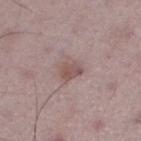Clinical impression:
Recorded during total-body skin imaging; not selected for excision or biopsy.
Acquisition and patient details:
A male subject aged around 50. On the leg. An algorithmic analysis of the crop reported about 9 CIELAB-L* units darker than the surrounding skin and a normalized border contrast of about 7. This is a white-light tile. A close-up tile cropped from a whole-body skin photograph, about 15 mm across.